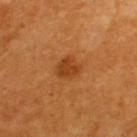notes: no biopsy performed (imaged during a skin exam) | subject: male, in their 60s | anatomic site: the upper back | image: 15 mm crop, total-body photography.